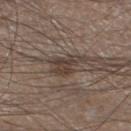biopsy_status: not biopsied; imaged during a skin examination
image:
  source: total-body photography crop
  field_of_view_mm: 15
lighting: white-light
site: leg
automated_metrics:
  area_mm2_approx: 5.0
  eccentricity: 0.75
  shape_asymmetry: 0.4
patient:
  sex: male
  age_approx: 45
lesion_size:
  long_diameter_mm_approx: 3.5